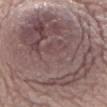Impression:
Captured during whole-body skin photography for melanoma surveillance; the lesion was not biopsied.
Image and clinical context:
A female subject roughly 65 years of age. A lesion tile, about 15 mm wide, cut from a 3D total-body photograph. Captured under white-light illumination. The lesion is on the chest. Approximately 14.5 mm at its widest.